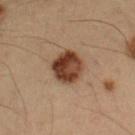<lesion>
<biopsy_status>not biopsied; imaged during a skin examination</biopsy_status>
<patient>
  <sex>male</sex>
  <age_approx>55</age_approx>
</patient>
<image>
  <source>total-body photography crop</source>
  <field_of_view_mm>15</field_of_view_mm>
</image>
<site>left forearm</site>
<automated_metrics>
  <vs_skin_darker_L>14.0</vs_skin_darker_L>
  <vs_skin_contrast_norm>12.5</vs_skin_contrast_norm>
  <border_irregularity_0_10>1.5</border_irregularity_0_10>
  <peripheral_color_asymmetry>2.5</peripheral_color_asymmetry>
</automated_metrics>
<lighting>cross-polarized</lighting>
</lesion>